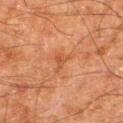The lesion was photographed on a routine skin check and not biopsied; there is no pathology result.
A roughly 15 mm field-of-view crop from a total-body skin photograph.
The recorded lesion diameter is about 3 mm.
Located on the right thigh.
The subject is a male aged approximately 80.
The tile uses cross-polarized illumination.